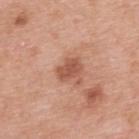<case>
<biopsy_status>not biopsied; imaged during a skin examination</biopsy_status>
<site>upper back</site>
<lighting>white-light</lighting>
<image>
  <source>total-body photography crop</source>
  <field_of_view_mm>15</field_of_view_mm>
</image>
<patient>
  <sex>female</sex>
  <age_approx>40</age_approx>
</patient>
</case>